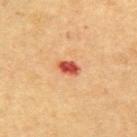{
  "biopsy_status": "not biopsied; imaged during a skin examination",
  "lighting": "cross-polarized",
  "automated_metrics": {
    "cielab_L": 49,
    "cielab_a": 31,
    "cielab_b": 34,
    "vs_skin_darker_L": 15.0,
    "vs_skin_contrast_norm": 10.0
  },
  "image": {
    "source": "total-body photography crop",
    "field_of_view_mm": 15
  },
  "patient": {
    "sex": "male",
    "age_approx": 60
  },
  "lesion_size": {
    "long_diameter_mm_approx": 3.0
  },
  "site": "arm"
}